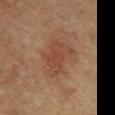Clinical impression: The lesion was tiled from a total-body skin photograph and was not biopsied. Background: The lesion is on the abdomen. Imaged with cross-polarized lighting. A roughly 15 mm field-of-view crop from a total-body skin photograph. A male patient aged approximately 65. Longest diameter approximately 5.5 mm.